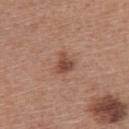<lesion>
<biopsy_status>not biopsied; imaged during a skin examination</biopsy_status>
<automated_metrics>
  <cielab_L>47</cielab_L>
  <cielab_a>23</cielab_a>
  <cielab_b>27</cielab_b>
  <vs_skin_darker_L>11.0</vs_skin_darker_L>
  <vs_skin_contrast_norm>8.0</vs_skin_contrast_norm>
</automated_metrics>
<lighting>white-light</lighting>
<image>
  <source>total-body photography crop</source>
  <field_of_view_mm>15</field_of_view_mm>
</image>
<lesion_size>
  <long_diameter_mm_approx>2.5</long_diameter_mm_approx>
</lesion_size>
<site>upper back</site>
<patient>
  <sex>female</sex>
  <age_approx>55</age_approx>
</patient>
</lesion>